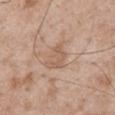No biopsy was performed on this lesion — it was imaged during a full skin examination and was not determined to be concerning. The total-body-photography lesion software estimated border irregularity of about 5.5 on a 0–10 scale, a color-variation rating of about 1.5/10, and peripheral color asymmetry of about 0.5. And it measured a detector confidence of about 100 out of 100 that the crop contains a lesion. A 15 mm close-up tile from a total-body photography series done for melanoma screening. A male patient aged 48 to 52. The tile uses white-light illumination. On the mid back.